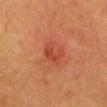image source: ~15 mm crop, total-body skin-cancer survey | size: about 3 mm | location: the head or neck | subject: female, aged 63 to 67 | tile lighting: cross-polarized illumination.